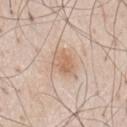location: the chest
acquisition: 15 mm crop, total-body photography
lesion diameter: ≈3.5 mm
subject: male, aged approximately 80
automated lesion analysis: a shape eccentricity near 0.65 and two-axis asymmetry of about 0.25; a border-irregularity rating of about 2.5/10, a within-lesion color-variation index near 3.5/10, and peripheral color asymmetry of about 1.5